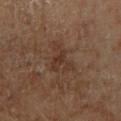{
  "biopsy_status": "not biopsied; imaged during a skin examination",
  "site": "right lower leg",
  "image": {
    "source": "total-body photography crop",
    "field_of_view_mm": 15
  },
  "patient": {
    "sex": "male",
    "age_approx": 70
  },
  "lighting": "cross-polarized",
  "lesion_size": {
    "long_diameter_mm_approx": 4.0
  }
}